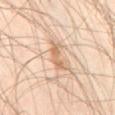| field | value |
|---|---|
| tile lighting | cross-polarized illumination |
| size | ≈3 mm |
| patient | male, roughly 45 years of age |
| TBP lesion metrics | an average lesion color of about L≈65 a*≈19 b*≈34 (CIELAB), a lesion–skin lightness drop of about 10, and a normalized border contrast of about 7 |
| imaging modality | ~15 mm tile from a whole-body skin photo |
| site | the lower back |